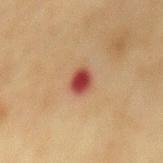The lesion was photographed on a routine skin check and not biopsied; there is no pathology result.
A 15 mm crop from a total-body photograph taken for skin-cancer surveillance.
Imaged with cross-polarized lighting.
Located on the mid back.
Automated image analysis of the tile measured internal color variation of about 3.5 on a 0–10 scale and peripheral color asymmetry of about 1. And it measured a classifier nevus-likeness of about 0/100 and lesion-presence confidence of about 100/100.
The lesion's longest dimension is about 3 mm.
A female subject, in their mid- to late 50s.